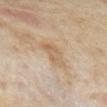Automated tile analysis of the lesion measured a lesion area of about 7 mm², a shape eccentricity near 0.85, and a shape-asymmetry score of about 0.35 (0 = symmetric). The analysis additionally found border irregularity of about 3.5 on a 0–10 scale, a within-lesion color-variation index near 3/10, and a peripheral color-asymmetry measure near 1. It also reported an automated nevus-likeness rating near 0 out of 100. A female patient approximately 35 years of age. From the left forearm. Imaged with cross-polarized lighting. A lesion tile, about 15 mm wide, cut from a 3D total-body photograph.